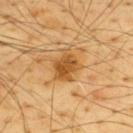{
  "biopsy_status": "not biopsied; imaged during a skin examination",
  "patient": {
    "sex": "male",
    "age_approx": 65
  },
  "lighting": "cross-polarized",
  "automated_metrics": {
    "eccentricity": 0.55,
    "shape_asymmetry": 0.35,
    "border_irregularity_0_10": 3.5,
    "color_variation_0_10": 4.5,
    "peripheral_color_asymmetry": 1.5
  },
  "site": "upper back",
  "image": {
    "source": "total-body photography crop",
    "field_of_view_mm": 15
  },
  "lesion_size": {
    "long_diameter_mm_approx": 3.5
  }
}